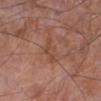A male subject, aged 58 to 62. The lesion is located on the right lower leg. This image is a 15 mm lesion crop taken from a total-body photograph.